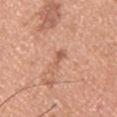biopsy status: imaged on a skin check; not biopsied
location: the front of the torso
automated metrics: an average lesion color of about L≈59 a*≈24 b*≈32 (CIELAB), a lesion–skin lightness drop of about 9, and a lesion-to-skin contrast of about 5.5 (normalized; higher = more distinct); a border-irregularity index near 4/10, internal color variation of about 1.5 on a 0–10 scale, and radial color variation of about 0.5; a nevus-likeness score of about 0/100 and lesion-presence confidence of about 100/100
lighting: white-light illumination
patient: male, aged 28–32
size: ~2.5 mm (longest diameter)
acquisition: 15 mm crop, total-body photography